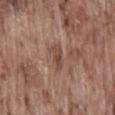Imaged with white-light lighting. From the lower back. Approximately 3 mm at its widest. A male patient aged approximately 75. This image is a 15 mm lesion crop taken from a total-body photograph.